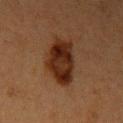This lesion was catalogued during total-body skin photography and was not selected for biopsy.
From the left upper arm.
Cropped from a total-body skin-imaging series; the visible field is about 15 mm.
About 5.5 mm across.
The subject is a female approximately 40 years of age.
Captured under cross-polarized illumination.